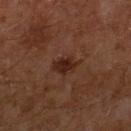A male subject, about 60 years old.
Located on the right lower leg.
A 15 mm crop from a total-body photograph taken for skin-cancer surveillance.